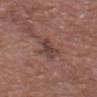Assessment:
The lesion was photographed on a routine skin check and not biopsied; there is no pathology result.
Background:
A region of skin cropped from a whole-body photographic capture, roughly 15 mm wide. Imaged with white-light lighting. The subject is a male aged approximately 80. On the chest.